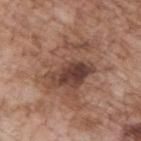Q: Was this lesion biopsied?
A: catalogued during a skin exam; not biopsied
Q: Who is the patient?
A: male, roughly 70 years of age
Q: Lesion size?
A: ~8.5 mm (longest diameter)
Q: What did automated image analysis measure?
A: a lesion area of about 33 mm² and a shape eccentricity near 0.7; roughly 10 lightness units darker than nearby skin and a normalized border contrast of about 7.5
Q: How was this image acquired?
A: 15 mm crop, total-body photography
Q: Lesion location?
A: the upper back